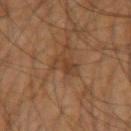Notes:
* acquisition: 15 mm crop, total-body photography
* illumination: cross-polarized
* site: the arm
* subject: male, about 60 years old
* automated metrics: a footprint of about 5 mm², an outline eccentricity of about 0.95 (0 = round, 1 = elongated), and a symmetry-axis asymmetry near 0.4; an average lesion color of about L≈36 a*≈17 b*≈28 (CIELAB), a lesion–skin lightness drop of about 6, and a normalized border contrast of about 5.5; an automated nevus-likeness rating near 0 out of 100 and lesion-presence confidence of about 100/100
* lesion diameter: ~4 mm (longest diameter)